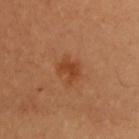| key | value |
|---|---|
| workup | no biopsy performed (imaged during a skin exam) |
| lesion size | ≈2.5 mm |
| location | the front of the torso |
| acquisition | ~15 mm tile from a whole-body skin photo |
| patient | female, about 50 years old |